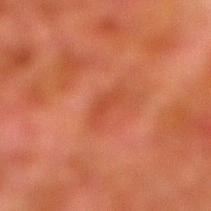This lesion was catalogued during total-body skin photography and was not selected for biopsy.
This image is a 15 mm lesion crop taken from a total-body photograph.
The lesion is on the leg.
The lesion-visualizer software estimated an area of roughly 2.5 mm² and a symmetry-axis asymmetry near 0.4. And it measured a mean CIELAB color near L≈37 a*≈28 b*≈32, roughly 5 lightness units darker than nearby skin, and a normalized border contrast of about 5. The software also gave a nevus-likeness score of about 0/100.
Longest diameter approximately 3 mm.
The patient is a male in their 80s.